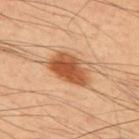Assessment:
No biopsy was performed on this lesion — it was imaged during a full skin examination and was not determined to be concerning.
Context:
Captured under cross-polarized illumination. The lesion-visualizer software estimated an outline eccentricity of about 0.8 (0 = round, 1 = elongated) and two-axis asymmetry of about 0.2. The analysis additionally found a border-irregularity rating of about 2.5/10. Longest diameter approximately 5 mm. A male subject, aged 58–62. Located on the upper back. Cropped from a whole-body photographic skin survey; the tile spans about 15 mm.